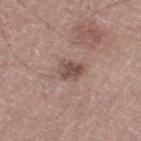| feature | finding |
|---|---|
| biopsy status | total-body-photography surveillance lesion; no biopsy |
| body site | the right thigh |
| image | total-body-photography crop, ~15 mm field of view |
| patient | male, in their mid-60s |